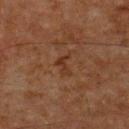{
  "biopsy_status": "not biopsied; imaged during a skin examination",
  "image": {
    "source": "total-body photography crop",
    "field_of_view_mm": 15
  },
  "lighting": "cross-polarized",
  "site": "upper back",
  "patient": {
    "sex": "male",
    "age_approx": 65
  }
}